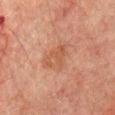<record>
  <image>
    <source>total-body photography crop</source>
    <field_of_view_mm>15</field_of_view_mm>
  </image>
  <patient>
    <sex>male</sex>
    <age_approx>75</age_approx>
  </patient>
  <site>chest</site>
</record>